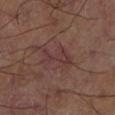- location — the left thigh
- lesion size — about 3.5 mm
- automated lesion analysis — a lesion area of about 5.5 mm² and a symmetry-axis asymmetry near 0.6; border irregularity of about 8 on a 0–10 scale, a color-variation rating of about 2/10, and peripheral color asymmetry of about 0.5; a nevus-likeness score of about 0/100 and a detector confidence of about 95 out of 100 that the crop contains a lesion
- illumination — cross-polarized illumination
- image source — ~15 mm tile from a whole-body skin photo